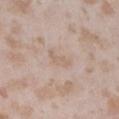Recorded during total-body skin imaging; not selected for excision or biopsy.